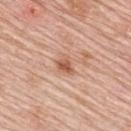Context:
A female patient, in their mid-60s. The tile uses white-light illumination. From the upper back. A close-up tile cropped from a whole-body skin photograph, about 15 mm across. The recorded lesion diameter is about 2.5 mm.